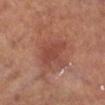| field | value |
|---|---|
| subject | male, approximately 70 years of age |
| site | the right lower leg |
| acquisition | ~15 mm crop, total-body skin-cancer survey |